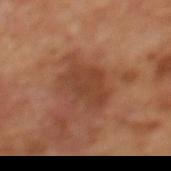Findings:
* patient · male, roughly 70 years of age
* imaging modality · total-body-photography crop, ~15 mm field of view
* lesion size · ≈6 mm
* location · the mid back
* tile lighting · cross-polarized